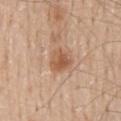Captured during whole-body skin photography for melanoma surveillance; the lesion was not biopsied. Located on the mid back. The subject is a male approximately 60 years of age. A 15 mm crop from a total-body photograph taken for skin-cancer surveillance. Measured at roughly 3.5 mm in maximum diameter.